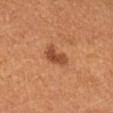Q: Is there a histopathology result?
A: catalogued during a skin exam; not biopsied
Q: What did automated image analysis measure?
A: a lesion area of about 5 mm², an outline eccentricity of about 0.75 (0 = round, 1 = elongated), and a shape-asymmetry score of about 0.3 (0 = symmetric); a nevus-likeness score of about 90/100 and a detector confidence of about 100 out of 100 that the crop contains a lesion
Q: What kind of image is this?
A: 15 mm crop, total-body photography
Q: How large is the lesion?
A: about 3 mm
Q: What is the anatomic site?
A: the left forearm
Q: What lighting was used for the tile?
A: cross-polarized
Q: What are the patient's age and sex?
A: female, aged 33–37